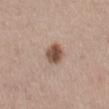Part of a total-body skin-imaging series; this lesion was reviewed on a skin check and was not flagged for biopsy. A lesion tile, about 15 mm wide, cut from a 3D total-body photograph. The lesion-visualizer software estimated a footprint of about 6 mm², a shape eccentricity near 0.5, and a shape-asymmetry score of about 0.15 (0 = symmetric). And it measured a border-irregularity rating of about 1.5/10 and a peripheral color-asymmetry measure near 1.5. The analysis additionally found a classifier nevus-likeness of about 95/100 and a lesion-detection confidence of about 100/100. The tile uses white-light illumination. A male subject, aged around 30. The lesion is located on the mid back.